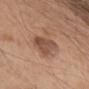Assessment: The lesion was tiled from a total-body skin photograph and was not biopsied. Background: From the left upper arm. Longest diameter approximately 6 mm. A 15 mm crop from a total-body photograph taken for skin-cancer surveillance. A male subject aged 63 to 67. This is a white-light tile.